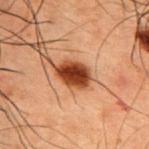Case summary:
* follow-up — no biopsy performed (imaged during a skin exam)
* imaging modality — ~15 mm crop, total-body skin-cancer survey
* tile lighting — cross-polarized illumination
* patient — male, roughly 50 years of age
* anatomic site — the upper back
* TBP lesion metrics — an area of roughly 7.5 mm², an outline eccentricity of about 0.75 (0 = round, 1 = elongated), and a shape-asymmetry score of about 0.15 (0 = symmetric); about 18 CIELAB-L* units darker than the surrounding skin and a lesion-to-skin contrast of about 15 (normalized; higher = more distinct); border irregularity of about 1.5 on a 0–10 scale, a color-variation rating of about 4/10, and a peripheral color-asymmetry measure near 1; a nevus-likeness score of about 100/100 and a detector confidence of about 100 out of 100 that the crop contains a lesion